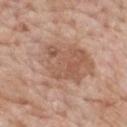Part of a total-body skin-imaging series; this lesion was reviewed on a skin check and was not flagged for biopsy.
The recorded lesion diameter is about 8 mm.
The subject is a male approximately 60 years of age.
The lesion is located on the mid back.
Cropped from a whole-body photographic skin survey; the tile spans about 15 mm.
The lesion-visualizer software estimated a lesion area of about 38 mm² and a shape-asymmetry score of about 0.3 (0 = symmetric). The analysis additionally found a border-irregularity rating of about 5.5/10, internal color variation of about 5 on a 0–10 scale, and a peripheral color-asymmetry measure near 1.5.